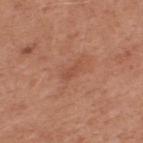<record>
<lesion_size>
  <long_diameter_mm_approx>2.5</long_diameter_mm_approx>
</lesion_size>
<automated_metrics>
  <border_irregularity_0_10>4.0</border_irregularity_0_10>
  <peripheral_color_asymmetry>0.0</peripheral_color_asymmetry>
</automated_metrics>
<image>
  <source>total-body photography crop</source>
  <field_of_view_mm>15</field_of_view_mm>
</image>
<patient>
  <sex>male</sex>
  <age_approx>55</age_approx>
</patient>
<site>upper back</site>
<lighting>white-light</lighting>
</record>